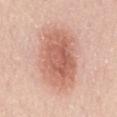The lesion is located on the back.
A male subject roughly 50 years of age.
This is a white-light tile.
A region of skin cropped from a whole-body photographic capture, roughly 15 mm wide.